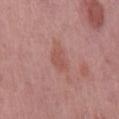Impression: Imaged during a routine full-body skin examination; the lesion was not biopsied and no histopathology is available. Acquisition and patient details: A male subject, aged 58 to 62. Located on the mid back. A lesion tile, about 15 mm wide, cut from a 3D total-body photograph.